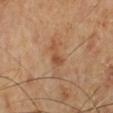biopsy status: no biopsy performed (imaged during a skin exam)
anatomic site: the leg
automated lesion analysis: an eccentricity of roughly 0.85 and a shape-asymmetry score of about 0.45 (0 = symmetric); a border-irregularity rating of about 5/10, a color-variation rating of about 1.5/10, and a peripheral color-asymmetry measure near 0.5
tile lighting: cross-polarized
patient: male, approximately 70 years of age
image source: ~15 mm crop, total-body skin-cancer survey
size: about 3 mm